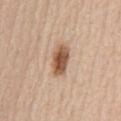Findings:
• follow-up: imaged on a skin check; not biopsied
• size: ~4 mm (longest diameter)
• image-analysis metrics: a mean CIELAB color near L≈55 a*≈20 b*≈32, roughly 16 lightness units darker than nearby skin, and a normalized border contrast of about 10.5; a border-irregularity rating of about 2/10, a color-variation rating of about 6.5/10, and radial color variation of about 2; a nevus-likeness score of about 100/100 and lesion-presence confidence of about 100/100
• subject: male, in their mid- to late 60s
• body site: the abdomen
• imaging modality: ~15 mm tile from a whole-body skin photo
• illumination: white-light